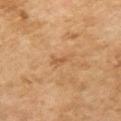A female subject about 60 years old.
From the chest.
This image is a 15 mm lesion crop taken from a total-body photograph.